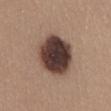notes: no biopsy performed (imaged during a skin exam)
location: the abdomen
patient: female, aged approximately 35
TBP lesion metrics: a lesion color around L≈38 a*≈17 b*≈20 in CIELAB and roughly 22 lightness units darker than nearby skin; a border-irregularity index near 1/10 and a within-lesion color-variation index near 6.5/10
acquisition: 15 mm crop, total-body photography
size: ≈5.5 mm